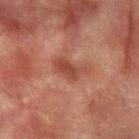The lesion was tiled from a total-body skin photograph and was not biopsied. Longest diameter approximately 4.5 mm. A male patient about 75 years old. Captured under cross-polarized illumination. Cropped from a whole-body photographic skin survey; the tile spans about 15 mm. On the left forearm. The lesion-visualizer software estimated a footprint of about 7 mm² and a symmetry-axis asymmetry near 0.4. And it measured a lesion color around L≈38 a*≈23 b*≈27 in CIELAB, about 8 CIELAB-L* units darker than the surrounding skin, and a lesion-to-skin contrast of about 7 (normalized; higher = more distinct). And it measured a nevus-likeness score of about 0/100 and a detector confidence of about 100 out of 100 that the crop contains a lesion.